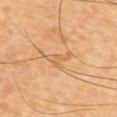This lesion was catalogued during total-body skin photography and was not selected for biopsy. Cropped from a total-body skin-imaging series; the visible field is about 15 mm. The lesion is located on the arm. A male subject approximately 60 years of age. Imaged with cross-polarized lighting.